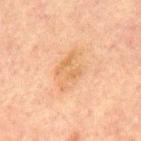{
  "biopsy_status": "not biopsied; imaged during a skin examination",
  "patient": {
    "sex": "male",
    "age_approx": 60
  },
  "image": {
    "source": "total-body photography crop",
    "field_of_view_mm": 15
  },
  "lesion_size": {
    "long_diameter_mm_approx": 5.0
  },
  "site": "back",
  "lighting": "cross-polarized"
}